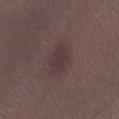follow-up: no biopsy performed (imaged during a skin exam) | automated metrics: an eccentricity of roughly 0.8; a normalized border contrast of about 6.5 | image: total-body-photography crop, ~15 mm field of view | body site: the left lower leg | size: about 4 mm | subject: male, about 55 years old | lighting: white-light illumination.